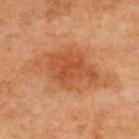workup = catalogued during a skin exam; not biopsied
acquisition = ~15 mm crop, total-body skin-cancer survey
lighting = cross-polarized
site = the back
subject = male, aged 68–72